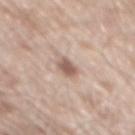Q: Was this lesion biopsied?
A: total-body-photography surveillance lesion; no biopsy
Q: What is the imaging modality?
A: ~15 mm crop, total-body skin-cancer survey
Q: Who is the patient?
A: male, approximately 80 years of age
Q: Automated lesion metrics?
A: roughly 13 lightness units darker than nearby skin and a normalized border contrast of about 8; a border-irregularity index near 1.5/10, a color-variation rating of about 2.5/10, and peripheral color asymmetry of about 1
Q: Where on the body is the lesion?
A: the mid back
Q: What is the lesion's diameter?
A: ~2.5 mm (longest diameter)